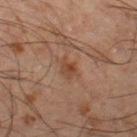Findings:
- biopsy status — total-body-photography surveillance lesion; no biopsy
- image source — 15 mm crop, total-body photography
- size — about 3 mm
- site — the left thigh
- patient — male, aged approximately 65
- illumination — cross-polarized illumination